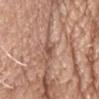Recorded during total-body skin imaging; not selected for excision or biopsy. A male patient, approximately 80 years of age. Cropped from a total-body skin-imaging series; the visible field is about 15 mm. The lesion is on the head or neck. The tile uses white-light illumination. The recorded lesion diameter is about 3 mm.